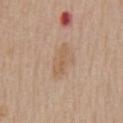Captured during whole-body skin photography for melanoma surveillance; the lesion was not biopsied. The tile uses white-light illumination. A lesion tile, about 15 mm wide, cut from a 3D total-body photograph. An algorithmic analysis of the crop reported an average lesion color of about L≈58 a*≈17 b*≈32 (CIELAB) and about 7 CIELAB-L* units darker than the surrounding skin. The software also gave a border-irregularity index near 4/10 and peripheral color asymmetry of about 0. The subject is a male aged around 65. From the chest. About 3.5 mm across.